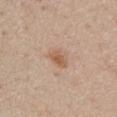Impression:
The lesion was tiled from a total-body skin photograph and was not biopsied.
Clinical summary:
From the chest. A male subject, roughly 75 years of age. Longest diameter approximately 2.5 mm. Captured under white-light illumination. A lesion tile, about 15 mm wide, cut from a 3D total-body photograph.